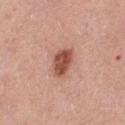Impression: The lesion was photographed on a routine skin check and not biopsied; there is no pathology result. Background: A male patient, in their mid- to late 50s. From the chest. The lesion's longest dimension is about 4 mm. A region of skin cropped from a whole-body photographic capture, roughly 15 mm wide.